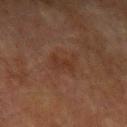The lesion was photographed on a routine skin check and not biopsied; there is no pathology result. The patient is a male approximately 75 years of age. The lesion's longest dimension is about 4 mm. A 15 mm crop from a total-body photograph taken for skin-cancer surveillance. The lesion is located on the left upper arm. The total-body-photography lesion software estimated a lesion area of about 6 mm² and a shape-asymmetry score of about 0.4 (0 = symmetric). The software also gave a classifier nevus-likeness of about 5/100. This is a cross-polarized tile.